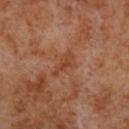Imaged during a routine full-body skin examination; the lesion was not biopsied and no histopathology is available.
The lesion is located on the left lower leg.
A male subject aged around 70.
Approximately 3.5 mm at its widest.
This image is a 15 mm lesion crop taken from a total-body photograph.
This is a cross-polarized tile.